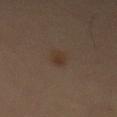Case summary:
- notes: total-body-photography surveillance lesion; no biopsy
- image-analysis metrics: a footprint of about 4 mm², an outline eccentricity of about 0.85 (0 = round, 1 = elongated), and two-axis asymmetry of about 0.3; a lesion color around L≈32 a*≈13 b*≈23 in CIELAB, a lesion–skin lightness drop of about 5, and a lesion-to-skin contrast of about 6 (normalized; higher = more distinct); a lesion-detection confidence of about 100/100
- image: ~15 mm tile from a whole-body skin photo
- lesion diameter: ~3 mm (longest diameter)
- location: the abdomen
- patient: male, approximately 70 years of age
- lighting: cross-polarized illumination